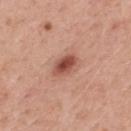{"biopsy_status": "not biopsied; imaged during a skin examination", "image": {"source": "total-body photography crop", "field_of_view_mm": 15}, "site": "mid back", "patient": {"sex": "male", "age_approx": 55}, "lighting": "white-light", "lesion_size": {"long_diameter_mm_approx": 3.5}, "automated_metrics": {"area_mm2_approx": 6.5, "eccentricity": 0.8, "shape_asymmetry": 0.25, "cielab_L": 51, "cielab_a": 26, "cielab_b": 28, "vs_skin_darker_L": 13.0, "vs_skin_contrast_norm": 9.0, "lesion_detection_confidence_0_100": 100}}